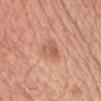Assessment:
The lesion was tiled from a total-body skin photograph and was not biopsied.
Background:
The recorded lesion diameter is about 3 mm. A roughly 15 mm field-of-view crop from a total-body skin photograph. A male patient, aged 53–57. From the head or neck. Automated tile analysis of the lesion measured a border-irregularity rating of about 3.5/10 and peripheral color asymmetry of about 1. The software also gave a nevus-likeness score of about 15/100 and a detector confidence of about 100 out of 100 that the crop contains a lesion.